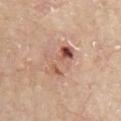No biopsy was performed on this lesion — it was imaged during a full skin examination and was not determined to be concerning.
The patient is a male aged around 65.
Measured at roughly 5 mm in maximum diameter.
The total-body-photography lesion software estimated a lesion area of about 10 mm², an eccentricity of roughly 0.85, and two-axis asymmetry of about 0.4. The software also gave border irregularity of about 5.5 on a 0–10 scale, a within-lesion color-variation index near 10/10, and radial color variation of about 4. The software also gave a classifier nevus-likeness of about 15/100 and a detector confidence of about 100 out of 100 that the crop contains a lesion.
A lesion tile, about 15 mm wide, cut from a 3D total-body photograph.
Captured under white-light illumination.
From the chest.